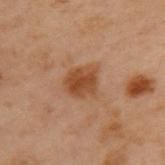Recorded during total-body skin imaging; not selected for excision or biopsy.
The tile uses cross-polarized illumination.
The subject is a male in their mid-50s.
The lesion-visualizer software estimated a mean CIELAB color near L≈38 a*≈19 b*≈29, a lesion–skin lightness drop of about 8, and a normalized lesion–skin contrast near 8. The analysis additionally found radial color variation of about 1. The analysis additionally found a nevus-likeness score of about 75/100.
The lesion is on the upper back.
This image is a 15 mm lesion crop taken from a total-body photograph.